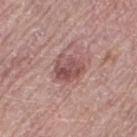{"biopsy_status": "not biopsied; imaged during a skin examination"}